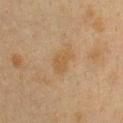Assessment:
This lesion was catalogued during total-body skin photography and was not selected for biopsy.
Background:
The subject is a female roughly 40 years of age. Located on the chest. Automated image analysis of the tile measured a border-irregularity rating of about 2.5/10 and peripheral color asymmetry of about 0.5. This is a cross-polarized tile. A 15 mm crop from a total-body photograph taken for skin-cancer surveillance.